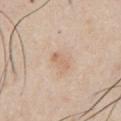subject: male, approximately 45 years of age | size: ≈3 mm | body site: the chest | image: 15 mm crop, total-body photography | automated metrics: a lesion color around L≈65 a*≈18 b*≈31 in CIELAB, a lesion–skin lightness drop of about 7, and a lesion-to-skin contrast of about 5 (normalized; higher = more distinct).